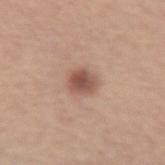| key | value |
|---|---|
| follow-up | no biopsy performed (imaged during a skin exam) |
| patient | female, approximately 65 years of age |
| image source | 15 mm crop, total-body photography |
| location | the arm |
| TBP lesion metrics | a detector confidence of about 100 out of 100 that the crop contains a lesion |
| tile lighting | white-light illumination |
| diameter | about 3 mm |